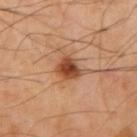The lesion was photographed on a routine skin check and not biopsied; there is no pathology result. Longest diameter approximately 3 mm. A 15 mm close-up extracted from a 3D total-body photography capture. Located on the right upper arm. This is a cross-polarized tile. Automated image analysis of the tile measured a footprint of about 6 mm², an outline eccentricity of about 0.3 (0 = round, 1 = elongated), and a shape-asymmetry score of about 0.25 (0 = symmetric). The software also gave a mean CIELAB color near L≈47 a*≈25 b*≈35 and roughly 15 lightness units darker than nearby skin. It also reported a border-irregularity rating of about 2/10 and radial color variation of about 1.5. The analysis additionally found a nevus-likeness score of about 100/100 and lesion-presence confidence of about 100/100. A male patient, in their 50s.